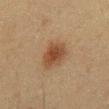<record>
  <biopsy_status>not biopsied; imaged during a skin examination</biopsy_status>
  <image>
    <source>total-body photography crop</source>
    <field_of_view_mm>15</field_of_view_mm>
  </image>
  <patient>
    <sex>male</sex>
    <age_approx>60</age_approx>
  </patient>
  <site>chest</site>
</record>